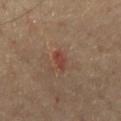From the chest. Cropped from a whole-body photographic skin survey; the tile spans about 15 mm. The recorded lesion diameter is about 3 mm. A male subject approximately 65 years of age. An algorithmic analysis of the crop reported a footprint of about 3.5 mm², an eccentricity of roughly 0.9, and a symmetry-axis asymmetry near 0.4. The tile uses cross-polarized illumination.